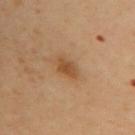Context: A female patient aged around 60. Imaged with cross-polarized lighting. A lesion tile, about 15 mm wide, cut from a 3D total-body photograph. Automated tile analysis of the lesion measured a lesion area of about 4 mm², an eccentricity of roughly 0.85, and two-axis asymmetry of about 0.2. The software also gave about 9 CIELAB-L* units darker than the surrounding skin and a normalized border contrast of about 8. The analysis additionally found a border-irregularity index near 2/10 and a peripheral color-asymmetry measure near 0.5. It also reported an automated nevus-likeness rating near 65 out of 100 and a detector confidence of about 100 out of 100 that the crop contains a lesion. The lesion is on the upper back.